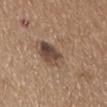follow-up: total-body-photography surveillance lesion; no biopsy | patient: male, about 65 years old | TBP lesion metrics: a lesion color around L≈50 a*≈15 b*≈27 in CIELAB and a lesion-to-skin contrast of about 6 (normalized; higher = more distinct) | anatomic site: the abdomen | imaging modality: 15 mm crop, total-body photography | lighting: white-light.